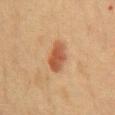Recorded during total-body skin imaging; not selected for excision or biopsy. Captured under cross-polarized illumination. This image is a 15 mm lesion crop taken from a total-body photograph. Automated image analysis of the tile measured a lesion area of about 8 mm² and a symmetry-axis asymmetry near 0.15. And it measured about 11 CIELAB-L* units darker than the surrounding skin. It also reported a border-irregularity index near 2/10 and a peripheral color-asymmetry measure near 1. The subject is a female in their 40s. On the front of the torso. Approximately 4.5 mm at its widest.